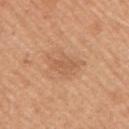Part of a total-body skin-imaging series; this lesion was reviewed on a skin check and was not flagged for biopsy.
The lesion's longest dimension is about 5 mm.
The subject is a male in their 50s.
The tile uses white-light illumination.
The lesion is located on the arm.
A lesion tile, about 15 mm wide, cut from a 3D total-body photograph.